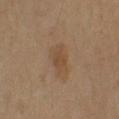<tbp_lesion>
  <site>right upper arm</site>
  <lesion_size>
    <long_diameter_mm_approx>3.5</long_diameter_mm_approx>
  </lesion_size>
  <lighting>cross-polarized</lighting>
  <patient>
    <sex>male</sex>
    <age_approx>55</age_approx>
  </patient>
  <image>
    <source>total-body photography crop</source>
    <field_of_view_mm>15</field_of_view_mm>
  </image>
</tbp_lesion>